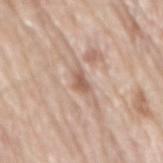site=the mid back | image=~15 mm tile from a whole-body skin photo | lesion size=~2.5 mm (longest diameter) | subject=male, aged 78 to 82 | lighting=white-light.